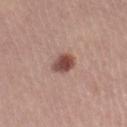On the right lower leg. This is a white-light tile. The patient is a female aged around 25. Measured at roughly 3 mm in maximum diameter. Cropped from a whole-body photographic skin survey; the tile spans about 15 mm.